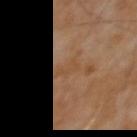biopsy status: catalogued during a skin exam; not biopsied
image source: 15 mm crop, total-body photography
size: ≈3 mm
subject: male, aged 63 to 67